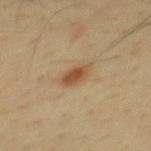follow-up: catalogued during a skin exam; not biopsied | imaging modality: ~15 mm crop, total-body skin-cancer survey | lesion size: ~3 mm (longest diameter) | location: the back | subject: male, in their mid-40s | tile lighting: cross-polarized illumination | automated metrics: a lesion area of about 5 mm², an eccentricity of roughly 0.8, and two-axis asymmetry of about 0.2; a mean CIELAB color near L≈49 a*≈18 b*≈34; a color-variation rating of about 2.5/10 and a peripheral color-asymmetry measure near 0.5; a lesion-detection confidence of about 100/100.